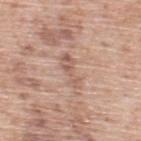| field | value |
|---|---|
| notes | total-body-photography surveillance lesion; no biopsy |
| acquisition | ~15 mm tile from a whole-body skin photo |
| lesion diameter | ~4.5 mm (longest diameter) |
| lighting | white-light |
| subject | male, aged around 55 |
| body site | the upper back |